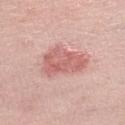Clinical summary: Imaged with white-light lighting. Located on the left lower leg. A female patient, in their mid-40s. The recorded lesion diameter is about 5.5 mm. This image is a 15 mm lesion crop taken from a total-body photograph.